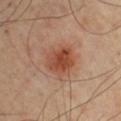Captured during whole-body skin photography for melanoma surveillance; the lesion was not biopsied. This is a cross-polarized tile. The recorded lesion diameter is about 4 mm. A male patient roughly 65 years of age. A lesion tile, about 15 mm wide, cut from a 3D total-body photograph. The lesion is on the arm.